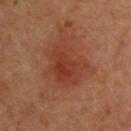biopsy status: imaged on a skin check; not biopsied | subject: female, about 50 years old | lesion diameter: about 5.5 mm | image source: total-body-photography crop, ~15 mm field of view | anatomic site: the upper back | automated metrics: about 7 CIELAB-L* units darker than the surrounding skin and a normalized lesion–skin contrast near 6.5; a border-irregularity index near 4/10, a within-lesion color-variation index near 4/10, and peripheral color asymmetry of about 1.